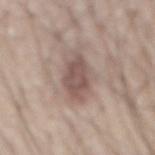Imaged during a routine full-body skin examination; the lesion was not biopsied and no histopathology is available. A close-up tile cropped from a whole-body skin photograph, about 15 mm across. The patient is a male aged around 75. From the mid back. Longest diameter approximately 6 mm. The lesion-visualizer software estimated a lesion area of about 13 mm², an eccentricity of roughly 0.85, and a shape-asymmetry score of about 0.2 (0 = symmetric).